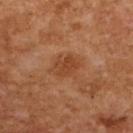No biopsy was performed on this lesion — it was imaged during a full skin examination and was not determined to be concerning.
Longest diameter approximately 3.5 mm.
Located on the upper back.
The tile uses cross-polarized illumination.
Cropped from a whole-body photographic skin survey; the tile spans about 15 mm.
A female subject in their 60s.